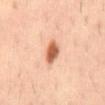Assessment:
This lesion was catalogued during total-body skin photography and was not selected for biopsy.
Acquisition and patient details:
A male patient, approximately 40 years of age. Cropped from a whole-body photographic skin survey; the tile spans about 15 mm. The lesion is located on the mid back.